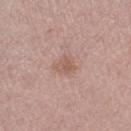illumination = white-light | TBP lesion metrics = an average lesion color of about L≈57 a*≈20 b*≈26 (CIELAB), a lesion–skin lightness drop of about 7, and a normalized lesion–skin contrast near 5.5 | subject = female, in their mid- to late 60s | body site = the right thigh | acquisition = 15 mm crop, total-body photography.